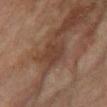Q: Patient demographics?
A: female, roughly 55 years of age
Q: How was the tile lit?
A: cross-polarized
Q: Lesion location?
A: the right thigh
Q: What is the lesion's diameter?
A: ~3 mm (longest diameter)
Q: What kind of image is this?
A: 15 mm crop, total-body photography